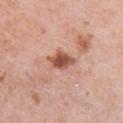follow-up = imaged on a skin check; not biopsied | patient = female, in their 40s | body site = the chest | imaging modality = ~15 mm crop, total-body skin-cancer survey | illumination = white-light illumination.